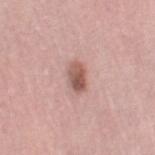Q: Is there a histopathology result?
A: imaged on a skin check; not biopsied
Q: Where on the body is the lesion?
A: the right thigh
Q: Patient demographics?
A: female, aged approximately 65
Q: How large is the lesion?
A: ≈3.5 mm
Q: What is the imaging modality?
A: total-body-photography crop, ~15 mm field of view
Q: How was the tile lit?
A: white-light